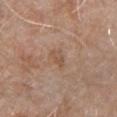Q: Was a biopsy performed?
A: no biopsy performed (imaged during a skin exam)
Q: Who is the patient?
A: male, about 80 years old
Q: How was this image acquired?
A: total-body-photography crop, ~15 mm field of view
Q: What is the anatomic site?
A: the chest
Q: What did automated image analysis measure?
A: a lesion area of about 3.5 mm² and an eccentricity of roughly 0.8; a border-irregularity rating of about 3.5/10, a within-lesion color-variation index near 2/10, and a peripheral color-asymmetry measure near 0.5
Q: What lighting was used for the tile?
A: white-light illumination
Q: Lesion size?
A: about 3 mm